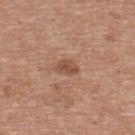workup — imaged on a skin check; not biopsied
body site — the upper back
acquisition — total-body-photography crop, ~15 mm field of view
subject — female, aged approximately 40